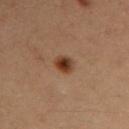Captured during whole-body skin photography for melanoma surveillance; the lesion was not biopsied. Imaged with cross-polarized lighting. The recorded lesion diameter is about 2.5 mm. A female subject in their mid- to late 30s. Automated tile analysis of the lesion measured a shape eccentricity near 0.65 and a symmetry-axis asymmetry near 0.2. And it measured an average lesion color of about L≈39 a*≈22 b*≈32 (CIELAB), roughly 13 lightness units darker than nearby skin, and a lesion-to-skin contrast of about 11 (normalized; higher = more distinct). And it measured a border-irregularity index near 2/10 and internal color variation of about 6.5 on a 0–10 scale. The lesion is located on the right upper arm. This image is a 15 mm lesion crop taken from a total-body photograph.